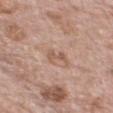Captured under white-light illumination. A region of skin cropped from a whole-body photographic capture, roughly 15 mm wide. An algorithmic analysis of the crop reported an area of roughly 2.5 mm² and an outline eccentricity of about 0.85 (0 = round, 1 = elongated). The software also gave a mean CIELAB color near L≈56 a*≈19 b*≈28 and roughly 8 lightness units darker than nearby skin. It also reported internal color variation of about 0 on a 0–10 scale and peripheral color asymmetry of about 0. The analysis additionally found a detector confidence of about 100 out of 100 that the crop contains a lesion. The patient is a female about 75 years old. Located on the chest.